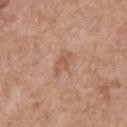The lesion was tiled from a total-body skin photograph and was not biopsied.
A male subject, aged around 80.
This is a white-light tile.
The total-body-photography lesion software estimated a footprint of about 5 mm² and an outline eccentricity of about 0.85 (0 = round, 1 = elongated). The software also gave a classifier nevus-likeness of about 0/100 and a detector confidence of about 100 out of 100 that the crop contains a lesion.
Cropped from a whole-body photographic skin survey; the tile spans about 15 mm.
From the upper back.
The recorded lesion diameter is about 3.5 mm.